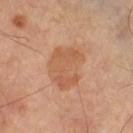{
  "biopsy_status": "not biopsied; imaged during a skin examination",
  "lighting": "cross-polarized",
  "automated_metrics": {
    "area_mm2_approx": 17.0,
    "shape_asymmetry": 0.2
  },
  "lesion_size": {
    "long_diameter_mm_approx": 5.5
  },
  "site": "right thigh",
  "image": {
    "source": "total-body photography crop",
    "field_of_view_mm": 15
  }
}